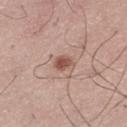No biopsy was performed on this lesion — it was imaged during a full skin examination and was not determined to be concerning. Automated tile analysis of the lesion measured a lesion area of about 4 mm² and an eccentricity of roughly 0.7. The analysis additionally found a mean CIELAB color near L≈53 a*≈21 b*≈26, roughly 12 lightness units darker than nearby skin, and a lesion-to-skin contrast of about 8 (normalized; higher = more distinct). It also reported a detector confidence of about 100 out of 100 that the crop contains a lesion. Longest diameter approximately 2.5 mm. Captured under white-light illumination. A 15 mm close-up extracted from a 3D total-body photography capture. A male subject, in their mid-50s.